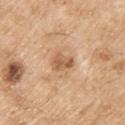Automated image analysis of the tile measured a footprint of about 4.5 mm², an eccentricity of roughly 0.7, and a symmetry-axis asymmetry near 0.35. The analysis additionally found a within-lesion color-variation index near 2.5/10 and peripheral color asymmetry of about 1. And it measured a classifier nevus-likeness of about 0/100 and a lesion-detection confidence of about 100/100. On the upper back. A 15 mm crop from a total-body photograph taken for skin-cancer surveillance. The patient is a male aged approximately 70. Longest diameter approximately 2.5 mm.